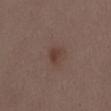A roughly 15 mm field-of-view crop from a total-body skin photograph.
Captured under white-light illumination.
The patient is a female aged approximately 30.
On the abdomen.
Measured at roughly 2.5 mm in maximum diameter.